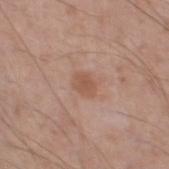Impression: Recorded during total-body skin imaging; not selected for excision or biopsy. Image and clinical context: Longest diameter approximately 2.5 mm. This image is a 15 mm lesion crop taken from a total-body photograph. Located on the left thigh. A male subject aged approximately 55.